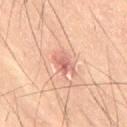follow-up=imaged on a skin check; not biopsied | anatomic site=the lower back | acquisition=total-body-photography crop, ~15 mm field of view | patient=male, in their 50s | tile lighting=cross-polarized illumination.